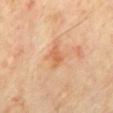Case summary:
* notes: imaged on a skin check; not biopsied
* illumination: cross-polarized
* patient: male, in their mid-60s
* image-analysis metrics: roughly 9 lightness units darker than nearby skin and a normalized border contrast of about 6; a border-irregularity rating of about 2.5/10 and internal color variation of about 3.5 on a 0–10 scale; an automated nevus-likeness rating near 0 out of 100 and a detector confidence of about 100 out of 100 that the crop contains a lesion
* image: total-body-photography crop, ~15 mm field of view
* body site: the mid back